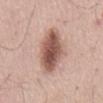The subject is a male in their mid- to late 50s.
The lesion is located on the abdomen.
A lesion tile, about 15 mm wide, cut from a 3D total-body photograph.